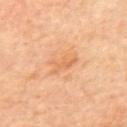Assessment: Captured during whole-body skin photography for melanoma surveillance; the lesion was not biopsied. Context: A 15 mm crop from a total-body photograph taken for skin-cancer surveillance. A male patient roughly 60 years of age. From the mid back. Approximately 3.5 mm at its widest.